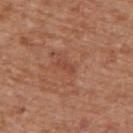{
  "biopsy_status": "not biopsied; imaged during a skin examination",
  "image": {
    "source": "total-body photography crop",
    "field_of_view_mm": 15
  },
  "lesion_size": {
    "long_diameter_mm_approx": 2.5
  },
  "patient": {
    "sex": "male",
    "age_approx": 65
  },
  "automated_metrics": {
    "border_irregularity_0_10": 4.0,
    "color_variation_0_10": 0.0
  },
  "lighting": "white-light",
  "site": "upper back"
}